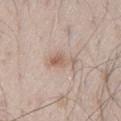Impression: The lesion was photographed on a routine skin check and not biopsied; there is no pathology result. Context: The patient is a male about 45 years old. Cropped from a total-body skin-imaging series; the visible field is about 15 mm. From the leg. Captured under white-light illumination. Measured at roughly 4 mm in maximum diameter.